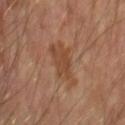follow-up: catalogued during a skin exam; not biopsied, diameter: ~5 mm (longest diameter), imaging modality: ~15 mm tile from a whole-body skin photo, location: the arm, patient: in their mid-50s.